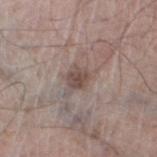Q: Was this lesion biopsied?
A: no biopsy performed (imaged during a skin exam)
Q: What is the imaging modality?
A: ~15 mm tile from a whole-body skin photo
Q: How large is the lesion?
A: about 2.5 mm
Q: What are the patient's age and sex?
A: male, roughly 55 years of age
Q: Illumination type?
A: white-light illumination
Q: What did automated image analysis measure?
A: a footprint of about 4.5 mm², an eccentricity of roughly 0.45, and a shape-asymmetry score of about 0.2 (0 = symmetric); a classifier nevus-likeness of about 40/100 and a lesion-detection confidence of about 100/100
Q: What is the anatomic site?
A: the left thigh